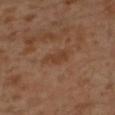biopsy status: no biopsy performed (imaged during a skin exam).